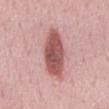follow-up: no biopsy performed (imaged during a skin exam)
subject: male, aged 58 to 62
image: total-body-photography crop, ~15 mm field of view
lesion diameter: ≈7.5 mm
anatomic site: the mid back
illumination: white-light illumination